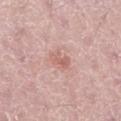About 2.5 mm across.
Captured under white-light illumination.
A male patient, aged 48 to 52.
The lesion is located on the left lower leg.
A 15 mm crop from a total-body photograph taken for skin-cancer surveillance.